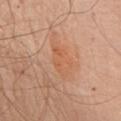Case summary:
• notes · imaged on a skin check; not biopsied
• TBP lesion metrics · a footprint of about 7 mm², an outline eccentricity of about 0.85 (0 = round, 1 = elongated), and a symmetry-axis asymmetry near 0.3
• subject · male, aged around 80
• lesion size · ≈4.5 mm
• image source · ~15 mm tile from a whole-body skin photo
• location · the chest
• lighting · white-light illumination